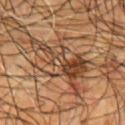body site — the chest; patient — male, roughly 55 years of age; imaging modality — total-body-photography crop, ~15 mm field of view; lesion size — ~9.5 mm (longest diameter); illumination — cross-polarized illumination.